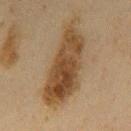biopsy_status: not biopsied; imaged during a skin examination
site: mid back
image:
  source: total-body photography crop
  field_of_view_mm: 15
lighting: cross-polarized
patient:
  sex: male
  age_approx: 60